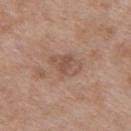{
  "biopsy_status": "not biopsied; imaged during a skin examination",
  "lesion_size": {
    "long_diameter_mm_approx": 4.0
  },
  "image": {
    "source": "total-body photography crop",
    "field_of_view_mm": 15
  },
  "site": "upper back",
  "automated_metrics": {
    "nevus_likeness_0_100": 0,
    "lesion_detection_confidence_0_100": 100
  },
  "patient": {
    "sex": "female",
    "age_approx": 40
  },
  "lighting": "white-light"
}